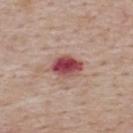Impression: Imaged during a routine full-body skin examination; the lesion was not biopsied and no histopathology is available. Acquisition and patient details: The lesion is on the upper back. The subject is a male aged approximately 75. This image is a 15 mm lesion crop taken from a total-body photograph. Measured at roughly 4 mm in maximum diameter. Captured under white-light illumination. Automated image analysis of the tile measured a border-irregularity rating of about 1.5/10, a within-lesion color-variation index near 8/10, and a peripheral color-asymmetry measure near 2.5. It also reported a classifier nevus-likeness of about 0/100 and a detector confidence of about 100 out of 100 that the crop contains a lesion.